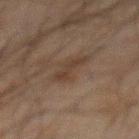{"site": "chest", "lighting": "cross-polarized", "image": {"source": "total-body photography crop", "field_of_view_mm": 15}, "patient": {"sex": "male", "age_approx": 60}}